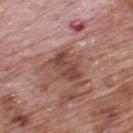Impression:
Imaged during a routine full-body skin examination; the lesion was not biopsied and no histopathology is available.
Acquisition and patient details:
The lesion's longest dimension is about 4 mm. The lesion is on the upper back. A 15 mm close-up tile from a total-body photography series done for melanoma screening. The patient is a male roughly 70 years of age.